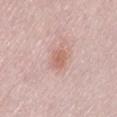Recorded during total-body skin imaging; not selected for excision or biopsy. The tile uses white-light illumination. From the lower back. The lesion's longest dimension is about 2.5 mm. A lesion tile, about 15 mm wide, cut from a 3D total-body photograph. The total-body-photography lesion software estimated a shape eccentricity near 0.65. The analysis additionally found a normalized border contrast of about 6.5. The analysis additionally found a classifier nevus-likeness of about 35/100 and lesion-presence confidence of about 100/100. A female subject, about 50 years old.